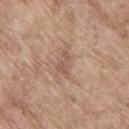Assessment: Captured during whole-body skin photography for melanoma surveillance; the lesion was not biopsied. Clinical summary: The lesion's longest dimension is about 3 mm. This is a white-light tile. A male patient, aged approximately 65. A region of skin cropped from a whole-body photographic capture, roughly 15 mm wide. From the upper back.